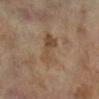workup — catalogued during a skin exam; not biopsied | illumination — cross-polarized | body site — the left lower leg | automated metrics — a footprint of about 11 mm², an eccentricity of roughly 0.9, and a symmetry-axis asymmetry near 0.2; a border-irregularity rating of about 3/10 and a peripheral color-asymmetry measure near 2.5; a lesion-detection confidence of about 100/100 | subject — female, about 60 years old | size — ~5.5 mm (longest diameter) | acquisition — 15 mm crop, total-body photography.